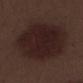Findings:
– biopsy status: total-body-photography surveillance lesion; no biopsy
– patient: male, in their 70s
– imaging modality: 15 mm crop, total-body photography
– anatomic site: the left lower leg